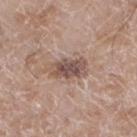notes — total-body-photography surveillance lesion; no biopsy | image — 15 mm crop, total-body photography | lesion size — ~5 mm (longest diameter) | TBP lesion metrics — a mean CIELAB color near L≈51 a*≈17 b*≈23 and a lesion-to-skin contrast of about 8.5 (normalized; higher = more distinct); a border-irregularity index near 3/10, a within-lesion color-variation index near 5/10, and radial color variation of about 1.5 | subject — male, aged around 60 | site — the left lower leg | illumination — white-light.